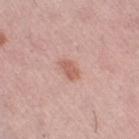Imaged during a routine full-body skin examination; the lesion was not biopsied and no histopathology is available.
The patient is a female aged 63–67.
The tile uses white-light illumination.
Automated image analysis of the tile measured a border-irregularity index near 3/10 and a within-lesion color-variation index near 1/10. It also reported a classifier nevus-likeness of about 70/100 and a detector confidence of about 100 out of 100 that the crop contains a lesion.
The lesion is located on the left thigh.
Measured at roughly 2.5 mm in maximum diameter.
A region of skin cropped from a whole-body photographic capture, roughly 15 mm wide.